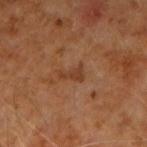The lesion was photographed on a routine skin check and not biopsied; there is no pathology result. The lesion-visualizer software estimated a mean CIELAB color near L≈37 a*≈21 b*≈31. It also reported peripheral color asymmetry of about 0.5. Located on the right upper arm. A region of skin cropped from a whole-body photographic capture, roughly 15 mm wide. A male patient about 60 years old. Imaged with cross-polarized lighting.